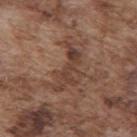<record>
  <biopsy_status>not biopsied; imaged during a skin examination</biopsy_status>
  <lesion_size>
    <long_diameter_mm_approx>6.0</long_diameter_mm_approx>
  </lesion_size>
  <site>upper back</site>
  <patient>
    <sex>male</sex>
    <age_approx>75</age_approx>
  </patient>
  <automated_metrics>
    <area_mm2_approx>13.0</area_mm2_approx>
    <eccentricity>0.85</eccentricity>
    <shape_asymmetry>0.55</shape_asymmetry>
    <border_irregularity_0_10>8.0</border_irregularity_0_10>
    <color_variation_0_10>5.5</color_variation_0_10>
    <peripheral_color_asymmetry>1.5</peripheral_color_asymmetry>
    <nevus_likeness_0_100>0</nevus_likeness_0_100>
  </automated_metrics>
  <image>
    <source>total-body photography crop</source>
    <field_of_view_mm>15</field_of_view_mm>
  </image>
  <lighting>white-light</lighting>
</record>